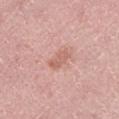Imaged during a routine full-body skin examination; the lesion was not biopsied and no histopathology is available.
Imaged with white-light lighting.
Automated image analysis of the tile measured internal color variation of about 2 on a 0–10 scale and radial color variation of about 0.5. The software also gave an automated nevus-likeness rating near 0 out of 100 and a lesion-detection confidence of about 100/100.
A female patient roughly 40 years of age.
The lesion is located on the leg.
A roughly 15 mm field-of-view crop from a total-body skin photograph.